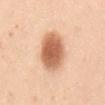Imaged during a routine full-body skin examination; the lesion was not biopsied and no histopathology is available.